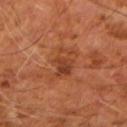biopsy status: no biopsy performed (imaged during a skin exam) | site: the right forearm | acquisition: ~15 mm crop, total-body skin-cancer survey | TBP lesion metrics: a lesion area of about 10 mm² and a shape eccentricity near 0.85; a mean CIELAB color near L≈40 a*≈26 b*≈34 and roughly 7 lightness units darker than nearby skin; a border-irregularity index near 4.5/10 and radial color variation of about 2.5; an automated nevus-likeness rating near 0 out of 100 | patient: male, about 60 years old.